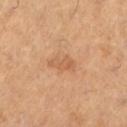<lesion>
  <biopsy_status>not biopsied; imaged during a skin examination</biopsy_status>
  <image>
    <source>total-body photography crop</source>
    <field_of_view_mm>15</field_of_view_mm>
  </image>
  <site>leg</site>
  <lighting>cross-polarized</lighting>
  <patient>
    <sex>female</sex>
    <age_approx>55</age_approx>
  </patient>
</lesion>